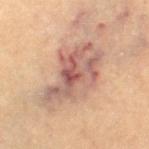Imaged during a routine full-body skin examination; the lesion was not biopsied and no histopathology is available. A region of skin cropped from a whole-body photographic capture, roughly 15 mm wide. The lesion-visualizer software estimated an area of roughly 28 mm², an outline eccentricity of about 0.85 (0 = round, 1 = elongated), and two-axis asymmetry of about 0.3. The analysis additionally found a mean CIELAB color near L≈58 a*≈20 b*≈26. And it measured a nevus-likeness score of about 5/100 and lesion-presence confidence of about 90/100. About 8.5 mm across. On the left leg. A female subject in their mid-60s.